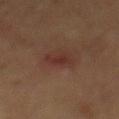notes = no biopsy performed (imaged during a skin exam) | subject = male, aged around 65 | image = total-body-photography crop, ~15 mm field of view | body site = the mid back.